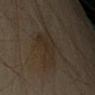Q: Was this lesion biopsied?
A: imaged on a skin check; not biopsied
Q: Lesion location?
A: the left upper arm
Q: How was the tile lit?
A: cross-polarized
Q: What are the patient's age and sex?
A: male, in their 60s
Q: What did automated image analysis measure?
A: an outline eccentricity of about 0.55 (0 = round, 1 = elongated) and two-axis asymmetry of about 0.3; a border-irregularity rating of about 4.5/10, a color-variation rating of about 3/10, and a peripheral color-asymmetry measure near 1; an automated nevus-likeness rating near 5 out of 100 and lesion-presence confidence of about 100/100
Q: What kind of image is this?
A: total-body-photography crop, ~15 mm field of view